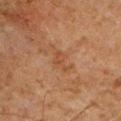Impression:
Recorded during total-body skin imaging; not selected for excision or biopsy.
Acquisition and patient details:
This image is a 15 mm lesion crop taken from a total-body photograph. The lesion is located on the front of the torso. About 3 mm across. Captured under cross-polarized illumination. A male subject aged 58 to 62.